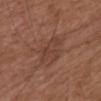A 15 mm crop from a total-body photograph taken for skin-cancer surveillance.
A male patient roughly 75 years of age.
This is a white-light tile.
The lesion's longest dimension is about 4 mm.
On the upper back.